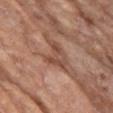follow-up: imaged on a skin check; not biopsied | location: the chest | lighting: white-light illumination | subject: female, in their mid- to late 70s | image: ~15 mm crop, total-body skin-cancer survey.